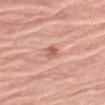{
  "biopsy_status": "not biopsied; imaged during a skin examination",
  "patient": {
    "sex": "female",
    "age_approx": 65
  },
  "image": {
    "source": "total-body photography crop",
    "field_of_view_mm": 15
  },
  "lesion_size": {
    "long_diameter_mm_approx": 2.5
  },
  "site": "left upper arm",
  "automated_metrics": {
    "area_mm2_approx": 3.0,
    "eccentricity": 0.75,
    "vs_skin_darker_L": 10.0,
    "vs_skin_contrast_norm": 7.0,
    "nevus_likeness_0_100": 5,
    "lesion_detection_confidence_0_100": 100
  }
}